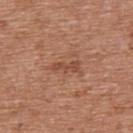Q: Is there a histopathology result?
A: total-body-photography surveillance lesion; no biopsy
Q: What is the imaging modality?
A: ~15 mm tile from a whole-body skin photo
Q: Lesion location?
A: the upper back
Q: Patient demographics?
A: male, approximately 65 years of age